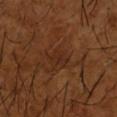biopsy_status: not biopsied; imaged during a skin examination
patient:
  sex: male
  age_approx: 65
automated_metrics:
  area_mm2_approx: 4.5
  eccentricity: 0.65
  shape_asymmetry: 0.3
  cielab_L: 27
  cielab_a: 21
  cielab_b: 29
  vs_skin_darker_L: 5.0
  vs_skin_contrast_norm: 5.5
lighting: cross-polarized
image:
  source: total-body photography crop
  field_of_view_mm: 15
site: right forearm
lesion_size:
  long_diameter_mm_approx: 2.5